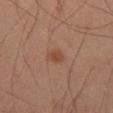Impression: Recorded during total-body skin imaging; not selected for excision or biopsy. Image and clinical context: The lesion-visualizer software estimated a lesion area of about 2.5 mm², an outline eccentricity of about 0.35 (0 = round, 1 = elongated), and a symmetry-axis asymmetry near 0.25. The analysis additionally found a lesion color around L≈45 a*≈21 b*≈31 in CIELAB and a normalized border contrast of about 6.5. And it measured a border-irregularity rating of about 2/10, internal color variation of about 1.5 on a 0–10 scale, and a peripheral color-asymmetry measure near 0.5. The analysis additionally found an automated nevus-likeness rating near 65 out of 100 and lesion-presence confidence of about 100/100. A male patient about 55 years old. A 15 mm crop from a total-body photograph taken for skin-cancer surveillance. Captured under cross-polarized illumination. From the right thigh.